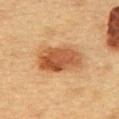diameter: ≈5.5 mm
acquisition: ~15 mm crop, total-body skin-cancer survey
site: the back
automated lesion analysis: an automated nevus-likeness rating near 100 out of 100 and a detector confidence of about 100 out of 100 that the crop contains a lesion
patient: female, aged approximately 60